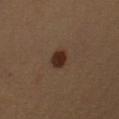{"lesion_size": {"long_diameter_mm_approx": 2.5}, "image": {"source": "total-body photography crop", "field_of_view_mm": 15}, "patient": {"sex": "female", "age_approx": 45}, "site": "left upper arm", "lighting": "cross-polarized"}